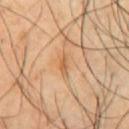Notes:
* notes — total-body-photography surveillance lesion; no biopsy
* subject — male, in their mid- to late 60s
* diameter — about 2.5 mm
* tile lighting — cross-polarized
* image-analysis metrics — a shape eccentricity near 0.9 and two-axis asymmetry of about 0.3; a detector confidence of about 100 out of 100 that the crop contains a lesion
* site — the front of the torso
* image source — ~15 mm crop, total-body skin-cancer survey